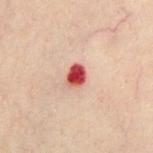Recorded during total-body skin imaging; not selected for excision or biopsy.
On the chest.
A male subject in their 50s.
A 15 mm close-up tile from a total-body photography series done for melanoma screening.